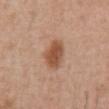Findings:
– biopsy status · total-body-photography surveillance lesion; no biopsy
– image · 15 mm crop, total-body photography
– lesion size · ~4 mm (longest diameter)
– illumination · white-light illumination
– body site · the abdomen
– patient · male, aged 53–57